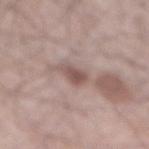automated lesion analysis: a footprint of about 4 mm² and a shape-asymmetry score of about 0.3 (0 = symmetric); an average lesion color of about L≈52 a*≈18 b*≈21 (CIELAB), roughly 11 lightness units darker than nearby skin, and a normalized border contrast of about 7.5
subject: male, in their mid- to late 60s
body site: the back
tile lighting: white-light
image source: 15 mm crop, total-body photography
lesion diameter: ~3 mm (longest diameter)